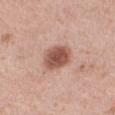follow-up — total-body-photography surveillance lesion; no biopsy | lesion size — about 3.5 mm | lighting — white-light | body site — the right thigh | subject — female, aged approximately 40 | image source — ~15 mm crop, total-body skin-cancer survey | TBP lesion metrics — an average lesion color of about L≈54 a*≈23 b*≈27 (CIELAB), about 14 CIELAB-L* units darker than the surrounding skin, and a normalized border contrast of about 9.5; an automated nevus-likeness rating near 100 out of 100 and a detector confidence of about 100 out of 100 that the crop contains a lesion.